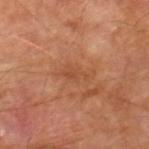biopsy status: no biopsy performed (imaged during a skin exam) | image: 15 mm crop, total-body photography | image-analysis metrics: a footprint of about 4.5 mm², a shape eccentricity near 0.9, and a shape-asymmetry score of about 0.4 (0 = symmetric); a border-irregularity index near 6.5/10 and internal color variation of about 1 on a 0–10 scale | subject: male, aged 68–72 | body site: the right lower leg | tile lighting: cross-polarized.